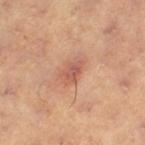Captured under cross-polarized illumination. A lesion tile, about 15 mm wide, cut from a 3D total-body photograph. Located on the left thigh. About 3 mm across. A female subject aged approximately 40.